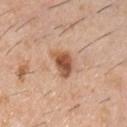| field | value |
|---|---|
| notes | no biopsy performed (imaged during a skin exam) |